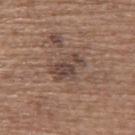Image and clinical context:
About 4 mm across. On the upper back. The patient is a female aged approximately 65. An algorithmic analysis of the crop reported a lesion area of about 7.5 mm² and a shape-asymmetry score of about 0.3 (0 = symmetric). It also reported a lesion color around L≈43 a*≈16 b*≈23 in CIELAB and a lesion–skin lightness drop of about 9. And it measured a border-irregularity index near 4.5/10 and internal color variation of about 4 on a 0–10 scale. The tile uses white-light illumination. A region of skin cropped from a whole-body photographic capture, roughly 15 mm wide.